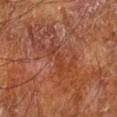biopsy_status: not biopsied; imaged during a skin examination
patient:
  sex: male
  age_approx: 65
lighting: cross-polarized
lesion_size:
  long_diameter_mm_approx: 4.5
image:
  source: total-body photography crop
  field_of_view_mm: 15
site: left lower leg